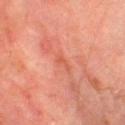The lesion was photographed on a routine skin check and not biopsied; there is no pathology result. A 15 mm close-up tile from a total-body photography series done for melanoma screening. The lesion is on the left lower leg. The subject is a male aged approximately 75.